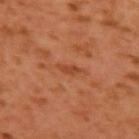biopsy status: catalogued during a skin exam; not biopsied
acquisition: 15 mm crop, total-body photography
subject: male, aged approximately 60
lighting: cross-polarized
anatomic site: the arm